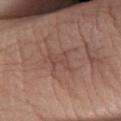Assessment: No biopsy was performed on this lesion — it was imaged during a full skin examination and was not determined to be concerning. Clinical summary: On the right lower leg. A 15 mm close-up extracted from a 3D total-body photography capture. This is a white-light tile. A female subject about 50 years old.